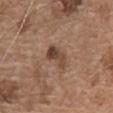Impression:
No biopsy was performed on this lesion — it was imaged during a full skin examination and was not determined to be concerning.
Clinical summary:
A male subject about 75 years old. A close-up tile cropped from a whole-body skin photograph, about 15 mm across. Located on the chest.